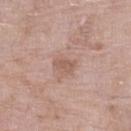{
  "site": "right lower leg",
  "lighting": "white-light",
  "patient": {
    "sex": "female",
    "age_approx": 75
  },
  "lesion_size": {
    "long_diameter_mm_approx": 2.5
  },
  "automated_metrics": {
    "area_mm2_approx": 3.0,
    "eccentricity": 0.8,
    "shape_asymmetry": 0.25,
    "border_irregularity_0_10": 2.5,
    "color_variation_0_10": 1.0,
    "nevus_likeness_0_100": 0,
    "lesion_detection_confidence_0_100": 100
  },
  "image": {
    "source": "total-body photography crop",
    "field_of_view_mm": 15
  }
}